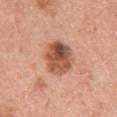Part of a total-body skin-imaging series; this lesion was reviewed on a skin check and was not flagged for biopsy. A female subject, about 40 years old. From the left upper arm. This image is a 15 mm lesion crop taken from a total-body photograph.